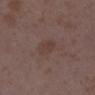Q: Illumination type?
A: white-light
Q: How large is the lesion?
A: ~3 mm (longest diameter)
Q: How was this image acquired?
A: ~15 mm crop, total-body skin-cancer survey
Q: What are the patient's age and sex?
A: female, aged 33–37
Q: Lesion location?
A: the right lower leg